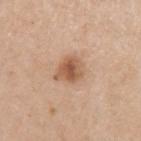  biopsy_status: not biopsied; imaged during a skin examination
  patient:
    sex: male
    age_approx: 60
  lighting: white-light
  lesion_size:
    long_diameter_mm_approx: 4.0
  site: left upper arm
  image:
    source: total-body photography crop
    field_of_view_mm: 15